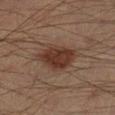biopsy status: no biopsy performed (imaged during a skin exam)
size: ≈5 mm
site: the right lower leg
illumination: cross-polarized illumination
image-analysis metrics: an area of roughly 11 mm², an eccentricity of roughly 0.75, and a symmetry-axis asymmetry near 0.2; an average lesion color of about L≈30 a*≈18 b*≈23 (CIELAB), a lesion–skin lightness drop of about 10, and a lesion-to-skin contrast of about 10 (normalized; higher = more distinct); a border-irregularity index near 2/10 and radial color variation of about 1
subject: male, in their 40s
acquisition: ~15 mm tile from a whole-body skin photo